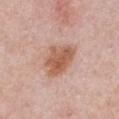Impression: Captured during whole-body skin photography for melanoma surveillance; the lesion was not biopsied. Acquisition and patient details: A female subject about 50 years old. Captured under white-light illumination. A lesion tile, about 15 mm wide, cut from a 3D total-body photograph. The lesion is located on the chest.